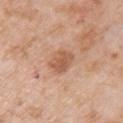The lesion was photographed on a routine skin check and not biopsied; there is no pathology result. The lesion is on the left upper arm. The subject is a female approximately 70 years of age. A 15 mm close-up extracted from a 3D total-body photography capture. Imaged with white-light lighting.